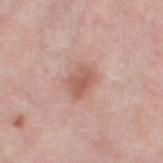<lesion>
  <biopsy_status>not biopsied; imaged during a skin examination</biopsy_status>
  <automated_metrics>
    <cielab_L>58</cielab_L>
    <cielab_a>23</cielab_a>
    <cielab_b>26</cielab_b>
    <vs_skin_darker_L>10.0</vs_skin_darker_L>
    <vs_skin_contrast_norm>6.5</vs_skin_contrast_norm>
    <border_irregularity_0_10>2.0</border_irregularity_0_10>
    <color_variation_0_10>3.5</color_variation_0_10>
    <nevus_likeness_0_100>30</nevus_likeness_0_100>
    <lesion_detection_confidence_0_100>100</lesion_detection_confidence_0_100>
  </automated_metrics>
  <lesion_size>
    <long_diameter_mm_approx>3.5</long_diameter_mm_approx>
  </lesion_size>
  <site>left thigh</site>
  <lighting>white-light</lighting>
  <image>
    <source>total-body photography crop</source>
    <field_of_view_mm>15</field_of_view_mm>
  </image>
  <patient>
    <sex>female</sex>
    <age_approx>40</age_approx>
  </patient>
</lesion>